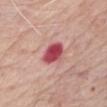Context: Located on the chest. Cropped from a whole-body photographic skin survey; the tile spans about 15 mm. Automated tile analysis of the lesion measured a lesion color around L≈50 a*≈37 b*≈22 in CIELAB, a lesion–skin lightness drop of about 18, and a normalized lesion–skin contrast near 11.5. Imaged with white-light lighting. A male subject, aged 78–82.